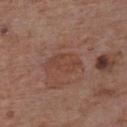Case summary:
– workup — catalogued during a skin exam; not biopsied
– lesion size — ≈4 mm
– illumination — white-light
– subject — male, about 60 years old
– body site — the chest
– image — ~15 mm tile from a whole-body skin photo
– image-analysis metrics — an area of roughly 6.5 mm², a shape eccentricity near 0.85, and a symmetry-axis asymmetry near 0.35; a lesion–skin lightness drop of about 6 and a normalized lesion–skin contrast near 5.5; border irregularity of about 4 on a 0–10 scale, a within-lesion color-variation index near 1.5/10, and peripheral color asymmetry of about 0.5; a classifier nevus-likeness of about 0/100 and lesion-presence confidence of about 100/100